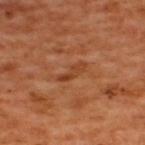Notes:
- notes · imaged on a skin check; not biopsied
- lighting · cross-polarized
- location · the back
- size · ~3.5 mm (longest diameter)
- acquisition · ~15 mm tile from a whole-body skin photo
- subject · male, approximately 50 years of age